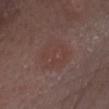No biopsy was performed on this lesion — it was imaged during a full skin examination and was not determined to be concerning.
A roughly 15 mm field-of-view crop from a total-body skin photograph.
Imaged with white-light lighting.
A female patient aged 48–52.
The lesion is located on the head or neck.
About 5 mm across.